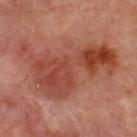Q: Is there a histopathology result?
A: no biopsy performed (imaged during a skin exam)
Q: Where on the body is the lesion?
A: the upper back
Q: What kind of image is this?
A: ~15 mm crop, total-body skin-cancer survey
Q: Illumination type?
A: cross-polarized illumination
Q: How large is the lesion?
A: about 11 mm
Q: What are the patient's age and sex?
A: male, roughly 50 years of age